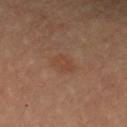Clinical impression:
No biopsy was performed on this lesion — it was imaged during a full skin examination and was not determined to be concerning.
Acquisition and patient details:
A female subject, roughly 70 years of age. The lesion is located on the right forearm. Automated tile analysis of the lesion measured a lesion area of about 4 mm², a shape eccentricity near 0.65, and a symmetry-axis asymmetry near 0.2. And it measured a border-irregularity rating of about 2/10 and a within-lesion color-variation index near 2.5/10. A 15 mm close-up tile from a total-body photography series done for melanoma screening.